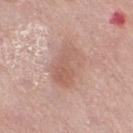follow-up: no biopsy performed (imaged during a skin exam) | location: the leg | automated metrics: an area of roughly 15 mm², an eccentricity of roughly 0.75, and a shape-asymmetry score of about 0.2 (0 = symmetric); a within-lesion color-variation index near 4/10 and a peripheral color-asymmetry measure near 1.5; a nevus-likeness score of about 5/100 and a detector confidence of about 100 out of 100 that the crop contains a lesion | patient: female, approximately 70 years of age | tile lighting: white-light illumination | imaging modality: 15 mm crop, total-body photography.